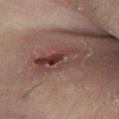Impression: Captured during whole-body skin photography for melanoma surveillance; the lesion was not biopsied. Clinical summary: The recorded lesion diameter is about 6.5 mm. Automated image analysis of the tile measured an area of roughly 13 mm² and a shape-asymmetry score of about 0.25 (0 = symmetric). And it measured a border-irregularity rating of about 4/10 and a peripheral color-asymmetry measure near 4.5. On the left lower leg. The tile uses cross-polarized illumination. A roughly 15 mm field-of-view crop from a total-body skin photograph. A female subject about 40 years old.